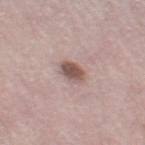Q: Was a biopsy performed?
A: imaged on a skin check; not biopsied
Q: How was this image acquired?
A: total-body-photography crop, ~15 mm field of view
Q: Who is the patient?
A: female, roughly 50 years of age
Q: Where on the body is the lesion?
A: the leg
Q: How was the tile lit?
A: white-light